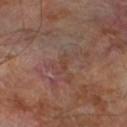No biopsy was performed on this lesion — it was imaged during a full skin examination and was not determined to be concerning. Longest diameter approximately 2.5 mm. Located on the left lower leg. Captured under cross-polarized illumination. Cropped from a total-body skin-imaging series; the visible field is about 15 mm. The subject is a male aged around 70. Automated image analysis of the tile measured a lesion–skin lightness drop of about 4 and a normalized lesion–skin contrast near 5. The analysis additionally found a border-irregularity rating of about 4/10, a within-lesion color-variation index near 0/10, and a peripheral color-asymmetry measure near 0.